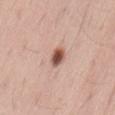The lesion-visualizer software estimated an area of roughly 4 mm², an outline eccentricity of about 0.7 (0 = round, 1 = elongated), and a shape-asymmetry score of about 0.3 (0 = symmetric). The analysis additionally found internal color variation of about 5 on a 0–10 scale and peripheral color asymmetry of about 1.5. The software also gave an automated nevus-likeness rating near 100 out of 100. The lesion is on the lower back. A male patient in their mid-30s. A 15 mm close-up tile from a total-body photography series done for melanoma screening.